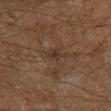notes: imaged on a skin check; not biopsied | lighting: cross-polarized | imaging modality: total-body-photography crop, ~15 mm field of view | anatomic site: the left lower leg | automated lesion analysis: a border-irregularity rating of about 3/10 and a color-variation rating of about 1.5/10 | lesion size: about 2.5 mm | patient: male, about 60 years old.